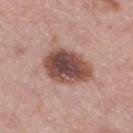Findings:
• notes · no biopsy performed (imaged during a skin exam)
• location · the left lower leg
• patient · female, approximately 50 years of age
• image · ~15 mm tile from a whole-body skin photo